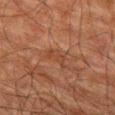| field | value |
|---|---|
| workup | total-body-photography surveillance lesion; no biopsy |
| patient | male, about 80 years old |
| image-analysis metrics | a lesion area of about 3.5 mm²; a mean CIELAB color near L≈34 a*≈19 b*≈27; a within-lesion color-variation index near 0.5/10 and peripheral color asymmetry of about 0.5 |
| lesion diameter | about 3 mm |
| acquisition | 15 mm crop, total-body photography |
| body site | the left thigh |
| tile lighting | cross-polarized illumination |